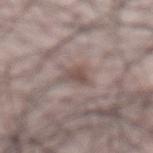Recorded during total-body skin imaging; not selected for excision or biopsy. About 2.5 mm across. A close-up tile cropped from a whole-body skin photograph, about 15 mm across. The lesion is on the abdomen. A male patient approximately 45 years of age. Imaged with white-light lighting.